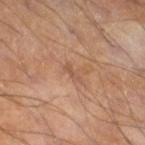Recorded during total-body skin imaging; not selected for excision or biopsy.
Captured under cross-polarized illumination.
The lesion is located on the right thigh.
This image is a 15 mm lesion crop taken from a total-body photograph.
A male subject, roughly 55 years of age.